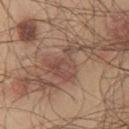The total-body-photography lesion software estimated a footprint of about 11 mm² and a shape-asymmetry score of about 0.65 (0 = symmetric). It also reported a mean CIELAB color near L≈48 a*≈19 b*≈25 and a normalized border contrast of about 6. The software also gave a border-irregularity index near 8/10, a color-variation rating of about 4/10, and peripheral color asymmetry of about 1. The lesion is located on the chest. A roughly 15 mm field-of-view crop from a total-body skin photograph. The subject is a male about 45 years old. Longest diameter approximately 5 mm.